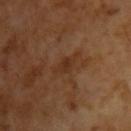The lesion was photographed on a routine skin check and not biopsied; there is no pathology result. A male subject in their mid- to late 60s. A region of skin cropped from a whole-body photographic capture, roughly 15 mm wide. The tile uses cross-polarized illumination. About 3.5 mm across.